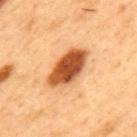Impression: Imaged during a routine full-body skin examination; the lesion was not biopsied and no histopathology is available. Context: From the mid back. This image is a 15 mm lesion crop taken from a total-body photograph. A male subject, aged approximately 50. The tile uses cross-polarized illumination. The recorded lesion diameter is about 6 mm. The total-body-photography lesion software estimated a border-irregularity index near 2/10, internal color variation of about 6.5 on a 0–10 scale, and radial color variation of about 1.5. It also reported a nevus-likeness score of about 100/100 and lesion-presence confidence of about 100/100.